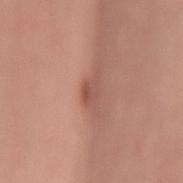Impression:
Captured during whole-body skin photography for melanoma surveillance; the lesion was not biopsied.
Background:
The tile uses white-light illumination. The lesion is on the lower back. The patient is a male about 65 years old. This image is a 15 mm lesion crop taken from a total-body photograph.